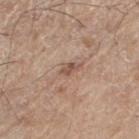Case summary:
– follow-up — total-body-photography surveillance lesion; no biopsy
– anatomic site — the left thigh
– patient — male, aged 68 to 72
– automated metrics — a lesion area of about 3 mm², a shape eccentricity near 0.85, and a shape-asymmetry score of about 0.2 (0 = symmetric); a lesion color around L≈53 a*≈18 b*≈27 in CIELAB; an automated nevus-likeness rating near 0 out of 100 and a detector confidence of about 100 out of 100 that the crop contains a lesion
– image source — ~15 mm crop, total-body skin-cancer survey